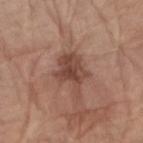acquisition: ~15 mm crop, total-body skin-cancer survey; illumination: white-light; image-analysis metrics: a within-lesion color-variation index near 3.5/10 and peripheral color asymmetry of about 1; patient: male, in their 70s; anatomic site: the right forearm.